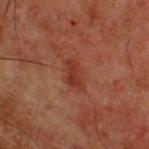Notes:
– follow-up · no biopsy performed (imaged during a skin exam)
– anatomic site · the upper back
– image-analysis metrics · a shape eccentricity near 0.8 and a symmetry-axis asymmetry near 0.3
– size · ~3 mm (longest diameter)
– acquisition · ~15 mm crop, total-body skin-cancer survey
– subject · male, aged approximately 50
– lighting · cross-polarized illumination